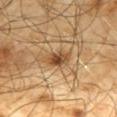workup: catalogued during a skin exam; not biopsied
TBP lesion metrics: a lesion area of about 5 mm², an eccentricity of roughly 0.85, and a symmetry-axis asymmetry near 0.2; an average lesion color of about L≈46 a*≈18 b*≈35 (CIELAB), about 12 CIELAB-L* units darker than the surrounding skin, and a lesion-to-skin contrast of about 9 (normalized; higher = more distinct); an automated nevus-likeness rating near 90 out of 100
diameter: ≈3.5 mm
subject: male, roughly 65 years of age
image source: total-body-photography crop, ~15 mm field of view
location: the mid back